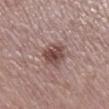Assessment: This lesion was catalogued during total-body skin photography and was not selected for biopsy. Clinical summary: Measured at roughly 3.5 mm in maximum diameter. The lesion is on the right lower leg. Imaged with white-light lighting. Cropped from a total-body skin-imaging series; the visible field is about 15 mm. The patient is a female in their mid-60s. Automated tile analysis of the lesion measured a lesion area of about 8 mm², an outline eccentricity of about 0.6 (0 = round, 1 = elongated), and two-axis asymmetry of about 0.2. It also reported a mean CIELAB color near L≈47 a*≈19 b*≈21 and a lesion-to-skin contrast of about 9 (normalized; higher = more distinct).